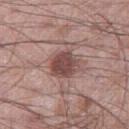This lesion was catalogued during total-body skin photography and was not selected for biopsy. The subject is a male aged 58–62. The lesion is located on the right thigh. Automated tile analysis of the lesion measured a mean CIELAB color near L≈47 a*≈20 b*≈21, about 13 CIELAB-L* units darker than the surrounding skin, and a normalized lesion–skin contrast near 9. A roughly 15 mm field-of-view crop from a total-body skin photograph. About 3.5 mm across. Captured under white-light illumination.